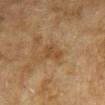Clinical impression:
Captured during whole-body skin photography for melanoma surveillance; the lesion was not biopsied.
Clinical summary:
A female subject approximately 55 years of age. Captured under cross-polarized illumination. Measured at roughly 2.5 mm in maximum diameter. An algorithmic analysis of the crop reported a footprint of about 3.5 mm² and an eccentricity of roughly 0.75. The software also gave an average lesion color of about L≈40 a*≈17 b*≈32 (CIELAB), a lesion–skin lightness drop of about 6, and a normalized lesion–skin contrast near 6. And it measured an automated nevus-likeness rating near 0 out of 100 and lesion-presence confidence of about 100/100. A 15 mm close-up tile from a total-body photography series done for melanoma screening. The lesion is on the right forearm.